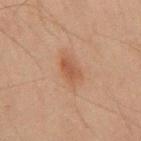Assessment: The lesion was tiled from a total-body skin photograph and was not biopsied. Context: Automated tile analysis of the lesion measured a classifier nevus-likeness of about 80/100 and a detector confidence of about 100 out of 100 that the crop contains a lesion. A male patient aged around 45. Measured at roughly 3 mm in maximum diameter. A roughly 15 mm field-of-view crop from a total-body skin photograph. Captured under cross-polarized illumination. From the mid back.